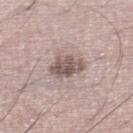| feature | finding |
|---|---|
| follow-up | no biopsy performed (imaged during a skin exam) |
| acquisition | ~15 mm crop, total-body skin-cancer survey |
| patient | male, approximately 35 years of age |
| illumination | white-light illumination |
| site | the left lower leg |